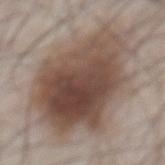Part of a total-body skin-imaging series; this lesion was reviewed on a skin check and was not flagged for biopsy. The lesion is located on the abdomen. A close-up tile cropped from a whole-body skin photograph, about 15 mm across. The tile uses white-light illumination. A male subject, approximately 55 years of age.